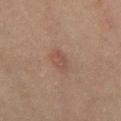{"biopsy_status": "not biopsied; imaged during a skin examination", "lesion_size": {"long_diameter_mm_approx": 2.5}, "site": "mid back", "patient": {"sex": "male", "age_approx": 70}, "lighting": "cross-polarized", "automated_metrics": {"shape_asymmetry": 0.25, "cielab_L": 40, "cielab_a": 16, "cielab_b": 21, "vs_skin_darker_L": 5.0, "vs_skin_contrast_norm": 5.0, "nevus_likeness_0_100": 15, "lesion_detection_confidence_0_100": 100}, "image": {"source": "total-body photography crop", "field_of_view_mm": 15}}